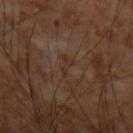Assessment:
Part of a total-body skin-imaging series; this lesion was reviewed on a skin check and was not flagged for biopsy.
Clinical summary:
The subject is a male about 55 years old. This is a cross-polarized tile. The lesion-visualizer software estimated a lesion area of about 2.5 mm² and an outline eccentricity of about 0.9 (0 = round, 1 = elongated). The analysis additionally found roughly 4 lightness units darker than nearby skin and a normalized border contrast of about 4.5. It also reported a border-irregularity index near 6.5/10, a color-variation rating of about 0/10, and a peripheral color-asymmetry measure near 0. It also reported an automated nevus-likeness rating near 0 out of 100. The lesion is on the left forearm. The lesion's longest dimension is about 3 mm. A 15 mm close-up extracted from a 3D total-body photography capture.